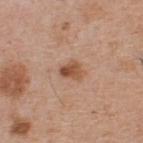Assessment: This lesion was catalogued during total-body skin photography and was not selected for biopsy. Background: Cropped from a whole-body photographic skin survey; the tile spans about 15 mm. Automated tile analysis of the lesion measured a footprint of about 4.5 mm², a shape eccentricity near 0.6, and two-axis asymmetry of about 0.25. The analysis additionally found a mean CIELAB color near L≈52 a*≈22 b*≈32 and a lesion-to-skin contrast of about 8 (normalized; higher = more distinct). It also reported border irregularity of about 2.5 on a 0–10 scale, a within-lesion color-variation index near 6/10, and radial color variation of about 2.5. A male patient approximately 60 years of age. From the back. The tile uses white-light illumination.